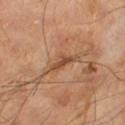subject = male, aged approximately 65
image = 15 mm crop, total-body photography